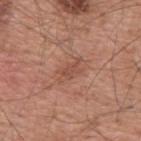notes = total-body-photography surveillance lesion; no biopsy
subject = male, aged 53 to 57
lesion diameter = ~3.5 mm (longest diameter)
imaging modality = ~15 mm tile from a whole-body skin photo
automated metrics = a border-irregularity index near 4.5/10 and a within-lesion color-variation index near 2.5/10
illumination = white-light illumination
site = the upper back